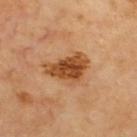Impression:
Recorded during total-body skin imaging; not selected for excision or biopsy.
Context:
The tile uses cross-polarized illumination. From the upper back. A close-up tile cropped from a whole-body skin photograph, about 15 mm across. The recorded lesion diameter is about 5 mm.